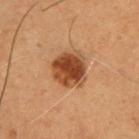Q: Was a biopsy performed?
A: imaged on a skin check; not biopsied
Q: What is the imaging modality?
A: 15 mm crop, total-body photography
Q: What did automated image analysis measure?
A: an average lesion color of about L≈36 a*≈21 b*≈30 (CIELAB)
Q: Where on the body is the lesion?
A: the chest
Q: Lesion size?
A: ~4 mm (longest diameter)
Q: How was the tile lit?
A: cross-polarized
Q: What are the patient's age and sex?
A: male, aged 48 to 52